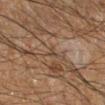Captured during whole-body skin photography for melanoma surveillance; the lesion was not biopsied. Approximately 1 mm at its widest. A close-up tile cropped from a whole-body skin photograph, about 15 mm across. The tile uses cross-polarized illumination. The lesion-visualizer software estimated a shape eccentricity near 0.7 and a shape-asymmetry score of about 0.35 (0 = symmetric). And it measured a mean CIELAB color near L≈39 a*≈14 b*≈25 and a normalized lesion–skin contrast near 5.5. And it measured a border-irregularity index near 3/10, a within-lesion color-variation index near 0/10, and radial color variation of about 0. The software also gave a nevus-likeness score of about 0/100 and a detector confidence of about 0 out of 100 that the crop contains a lesion. A male patient, roughly 60 years of age. On the right lower leg.